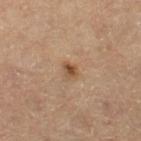Imaged during a routine full-body skin examination; the lesion was not biopsied and no histopathology is available. A female patient in their mid- to late 60s. This is a cross-polarized tile. Automated image analysis of the tile measured an average lesion color of about L≈49 a*≈19 b*≈33 (CIELAB), about 11 CIELAB-L* units darker than the surrounding skin, and a normalized border contrast of about 8. And it measured border irregularity of about 2 on a 0–10 scale and a peripheral color-asymmetry measure near 1.5. The lesion is located on the leg. Cropped from a whole-body photographic skin survey; the tile spans about 15 mm. Longest diameter approximately 2 mm.